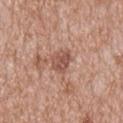Q: Was this lesion biopsied?
A: total-body-photography surveillance lesion; no biopsy
Q: How was this image acquired?
A: ~15 mm crop, total-body skin-cancer survey
Q: What are the patient's age and sex?
A: male, aged 63–67
Q: Automated lesion metrics?
A: an average lesion color of about L≈52 a*≈22 b*≈27 (CIELAB), roughly 10 lightness units darker than nearby skin, and a normalized lesion–skin contrast near 7; a border-irregularity rating of about 2.5/10 and a peripheral color-asymmetry measure near 1; lesion-presence confidence of about 100/100
Q: Where on the body is the lesion?
A: the mid back